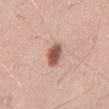notes: imaged on a skin check; not biopsied | image source: total-body-photography crop, ~15 mm field of view | site: the abdomen | subject: female, in their mid- to late 60s | tile lighting: white-light | size: about 3 mm.